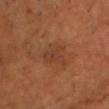Q: Is there a histopathology result?
A: imaged on a skin check; not biopsied
Q: Where on the body is the lesion?
A: the chest
Q: Illumination type?
A: cross-polarized illumination
Q: What did automated image analysis measure?
A: a nevus-likeness score of about 0/100 and a lesion-detection confidence of about 100/100
Q: How large is the lesion?
A: about 3.5 mm
Q: Who is the patient?
A: male, in their mid-60s
Q: What is the imaging modality?
A: 15 mm crop, total-body photography